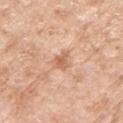Part of a total-body skin-imaging series; this lesion was reviewed on a skin check and was not flagged for biopsy. A female patient in their mid- to late 70s. From the left upper arm. A lesion tile, about 15 mm wide, cut from a 3D total-body photograph. Longest diameter approximately 3.5 mm. This is a white-light tile.